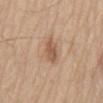| key | value |
|---|---|
| biopsy status | no biopsy performed (imaged during a skin exam) |
| patient | male, aged 78 to 82 |
| site | the mid back |
| lighting | white-light |
| diameter | ≈3.5 mm |
| imaging modality | total-body-photography crop, ~15 mm field of view |